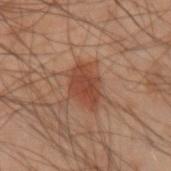Assessment:
No biopsy was performed on this lesion — it was imaged during a full skin examination and was not determined to be concerning.
Context:
The lesion is on the left arm. A 15 mm close-up tile from a total-body photography series done for melanoma screening. The patient is a male about 50 years old.